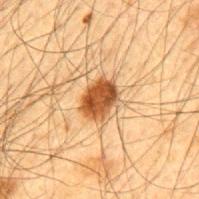Impression: Recorded during total-body skin imaging; not selected for excision or biopsy. Image and clinical context: An algorithmic analysis of the crop reported a border-irregularity rating of about 2/10 and a within-lesion color-variation index near 4/10. The analysis additionally found an automated nevus-likeness rating near 100 out of 100 and lesion-presence confidence of about 100/100. A 15 mm close-up tile from a total-body photography series done for melanoma screening. Captured under cross-polarized illumination. The lesion is located on the mid back. Approximately 4 mm at its widest. The patient is a male aged 63–67.